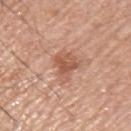{"biopsy_status": "not biopsied; imaged during a skin examination", "site": "upper back", "patient": {"sex": "male", "age_approx": 55}, "automated_metrics": {"area_mm2_approx": 7.5, "eccentricity": 0.55, "shape_asymmetry": 0.4, "border_irregularity_0_10": 4.0, "color_variation_0_10": 3.0, "peripheral_color_asymmetry": 1.0}, "image": {"source": "total-body photography crop", "field_of_view_mm": 15}, "lighting": "white-light"}